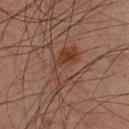Captured during whole-body skin photography for melanoma surveillance; the lesion was not biopsied. Longest diameter approximately 5.5 mm. The lesion is on the chest. This is a cross-polarized tile. A male patient in their mid- to late 30s. A roughly 15 mm field-of-view crop from a total-body skin photograph. The total-body-photography lesion software estimated a nevus-likeness score of about 95/100 and lesion-presence confidence of about 100/100.